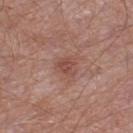biopsy_status: not biopsied; imaged during a skin examination
patient:
  sex: male
  age_approx: 55
lesion_size:
  long_diameter_mm_approx: 2.5
image:
  source: total-body photography crop
  field_of_view_mm: 15
site: leg